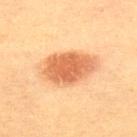Q: Was a biopsy performed?
A: catalogued during a skin exam; not biopsied
Q: Automated lesion metrics?
A: a lesion area of about 21 mm² and two-axis asymmetry of about 0.15; a lesion–skin lightness drop of about 13 and a lesion-to-skin contrast of about 8 (normalized; higher = more distinct); border irregularity of about 1.5 on a 0–10 scale, internal color variation of about 4.5 on a 0–10 scale, and a peripheral color-asymmetry measure near 1; an automated nevus-likeness rating near 100 out of 100
Q: Lesion location?
A: the upper back
Q: How was this image acquired?
A: ~15 mm tile from a whole-body skin photo
Q: Lesion size?
A: ~7 mm (longest diameter)
Q: What are the patient's age and sex?
A: female, aged 63–67
Q: Illumination type?
A: cross-polarized illumination